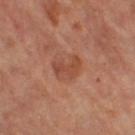Imaged during a routine full-body skin examination; the lesion was not biopsied and no histopathology is available. A lesion tile, about 15 mm wide, cut from a 3D total-body photograph. The tile uses cross-polarized illumination. On the left leg. Longest diameter approximately 3.5 mm. A female patient, in their mid-60s.